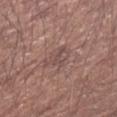{
  "biopsy_status": "not biopsied; imaged during a skin examination",
  "site": "left upper arm",
  "image": {
    "source": "total-body photography crop",
    "field_of_view_mm": 15
  },
  "patient": {
    "sex": "male",
    "age_approx": 65
  },
  "lighting": "white-light",
  "lesion_size": {
    "long_diameter_mm_approx": 3.5
  }
}